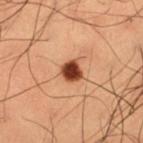Findings:
• biopsy status: imaged on a skin check; not biopsied